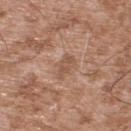Q: Is there a histopathology result?
A: no biopsy performed (imaged during a skin exam)
Q: Lesion size?
A: ≈3 mm
Q: What kind of image is this?
A: ~15 mm tile from a whole-body skin photo
Q: Who is the patient?
A: male, aged approximately 55
Q: Illumination type?
A: white-light
Q: Where on the body is the lesion?
A: the upper back
Q: What did automated image analysis measure?
A: roughly 8 lightness units darker than nearby skin; a color-variation rating of about 1.5/10 and radial color variation of about 0.5; a lesion-detection confidence of about 100/100